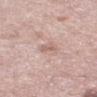Imaged during a routine full-body skin examination; the lesion was not biopsied and no histopathology is available. Approximately 2.5 mm at its widest. A female subject aged around 50. Cropped from a total-body skin-imaging series; the visible field is about 15 mm. Located on the left thigh.